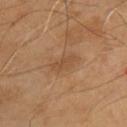This lesion was catalogued during total-body skin photography and was not selected for biopsy.
The lesion is located on the chest.
This is a cross-polarized tile.
Approximately 3.5 mm at its widest.
Cropped from a whole-body photographic skin survey; the tile spans about 15 mm.
A male patient, in their mid-50s.